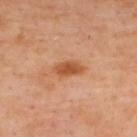<tbp_lesion>
  <biopsy_status>not biopsied; imaged during a skin examination</biopsy_status>
  <lighting>cross-polarized</lighting>
  <automated_metrics>
    <area_mm2_approx>6.0</area_mm2_approx>
    <eccentricity>0.85</eccentricity>
    <nevus_likeness_0_100>90</nevus_likeness_0_100>
    <lesion_detection_confidence_0_100>100</lesion_detection_confidence_0_100>
  </automated_metrics>
  <image>
    <source>total-body photography crop</source>
    <field_of_view_mm>15</field_of_view_mm>
  </image>
  <site>upper back</site>
  <patient>
    <sex>female</sex>
    <age_approx>40</age_approx>
  </patient>
  <lesion_size>
    <long_diameter_mm_approx>4.0</long_diameter_mm_approx>
  </lesion_size>
</tbp_lesion>